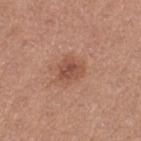Cropped from a total-body skin-imaging series; the visible field is about 15 mm.
On the left thigh.
The patient is a female about 50 years old.
Measured at roughly 3 mm in maximum diameter.